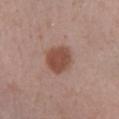Assessment:
No biopsy was performed on this lesion — it was imaged during a full skin examination and was not determined to be concerning.
Acquisition and patient details:
The lesion's longest dimension is about 4 mm. Located on the left lower leg. A female patient, aged around 50. A lesion tile, about 15 mm wide, cut from a 3D total-body photograph. The total-body-photography lesion software estimated a footprint of about 10 mm², an outline eccentricity of about 0.4 (0 = round, 1 = elongated), and a symmetry-axis asymmetry near 0.15. The software also gave an average lesion color of about L≈48 a*≈21 b*≈27 (CIELAB) and a normalized border contrast of about 9. The tile uses white-light illumination.